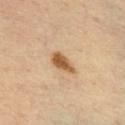Imaged during a routine full-body skin examination; the lesion was not biopsied and no histopathology is available. The subject is a male aged around 35. Automated image analysis of the tile measured a within-lesion color-variation index near 2.5/10 and a peripheral color-asymmetry measure near 1. Measured at roughly 3.5 mm in maximum diameter. A region of skin cropped from a whole-body photographic capture, roughly 15 mm wide. This is a cross-polarized tile. The lesion is on the front of the torso.